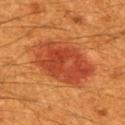Clinical impression: Captured during whole-body skin photography for melanoma surveillance; the lesion was not biopsied.